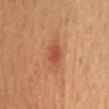A male subject, aged around 60.
The lesion's longest dimension is about 3 mm.
Cropped from a whole-body photographic skin survey; the tile spans about 15 mm.
The lesion is on the abdomen.